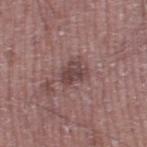<tbp_lesion>
  <biopsy_status>not biopsied; imaged during a skin examination</biopsy_status>
  <site>left thigh</site>
  <patient>
    <sex>male</sex>
    <age_approx>65</age_approx>
  </patient>
  <image>
    <source>total-body photography crop</source>
    <field_of_view_mm>15</field_of_view_mm>
  </image>
</tbp_lesion>